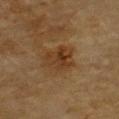The patient is a male in their mid- to late 80s or older. The lesion is on the chest. Longest diameter approximately 4 mm. An algorithmic analysis of the crop reported a lesion area of about 9.5 mm², a shape eccentricity near 0.65, and two-axis asymmetry of about 0.3. The analysis additionally found roughly 7 lightness units darker than nearby skin and a lesion-to-skin contrast of about 7 (normalized; higher = more distinct). It also reported a border-irregularity rating of about 3/10, internal color variation of about 4.5 on a 0–10 scale, and a peripheral color-asymmetry measure near 1.5. A close-up tile cropped from a whole-body skin photograph, about 15 mm across.